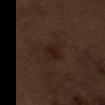Notes:
– subject: male, aged 68–72
– acquisition: total-body-photography crop, ~15 mm field of view
– site: the left upper arm
– automated lesion analysis: internal color variation of about 3.5 on a 0–10 scale and peripheral color asymmetry of about 1; an automated nevus-likeness rating near 35 out of 100 and lesion-presence confidence of about 100/100
– lighting: white-light